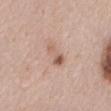Notes:
• workup · no biopsy performed (imaged during a skin exam)
• tile lighting · white-light
• subject · female, aged around 40
• image source · ~15 mm crop, total-body skin-cancer survey
• lesion size · ~3 mm (longest diameter)
• location · the mid back